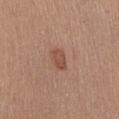Assessment:
The lesion was photographed on a routine skin check and not biopsied; there is no pathology result.
Acquisition and patient details:
The lesion's longest dimension is about 2.5 mm. Automated image analysis of the tile measured a border-irregularity rating of about 2.5/10, internal color variation of about 2.5 on a 0–10 scale, and radial color variation of about 1. The analysis additionally found a nevus-likeness score of about 65/100 and a detector confidence of about 100 out of 100 that the crop contains a lesion. A lesion tile, about 15 mm wide, cut from a 3D total-body photograph. The lesion is located on the abdomen. A female subject, aged 53–57.